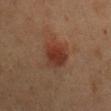The lesion was tiled from a total-body skin photograph and was not biopsied. A female subject roughly 40 years of age. The lesion's longest dimension is about 4.5 mm. The lesion is located on the mid back. A region of skin cropped from a whole-body photographic capture, roughly 15 mm wide. Captured under cross-polarized illumination. The lesion-visualizer software estimated about 8 CIELAB-L* units darker than the surrounding skin and a lesion-to-skin contrast of about 8 (normalized; higher = more distinct). The software also gave an automated nevus-likeness rating near 85 out of 100 and a detector confidence of about 100 out of 100 that the crop contains a lesion.